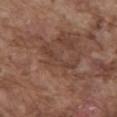Findings:
- biopsy status: total-body-photography surveillance lesion; no biopsy
- image: ~15 mm crop, total-body skin-cancer survey
- TBP lesion metrics: a border-irregularity rating of about 8/10, a within-lesion color-variation index near 1.5/10, and peripheral color asymmetry of about 0.5; a nevus-likeness score of about 0/100 and a lesion-detection confidence of about 95/100
- illumination: white-light
- site: the abdomen
- subject: male, aged around 75
- lesion size: ~5.5 mm (longest diameter)